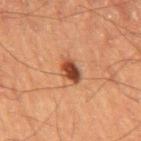Findings:
– biopsy status · catalogued during a skin exam; not biopsied
– image-analysis metrics · a footprint of about 5 mm² and an outline eccentricity of about 0.6 (0 = round, 1 = elongated); about 15 CIELAB-L* units darker than the surrounding skin and a lesion-to-skin contrast of about 11 (normalized; higher = more distinct)
– diameter · about 3 mm
– lighting · cross-polarized
– subject · male, aged 58 to 62
– image · total-body-photography crop, ~15 mm field of view